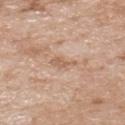<lesion>
<biopsy_status>not biopsied; imaged during a skin examination</biopsy_status>
<lighting>white-light</lighting>
<lesion_size>
  <long_diameter_mm_approx>3.0</long_diameter_mm_approx>
</lesion_size>
<site>upper back</site>
<image>
  <source>total-body photography crop</source>
  <field_of_view_mm>15</field_of_view_mm>
</image>
<patient>
  <sex>male</sex>
  <age_approx>80</age_approx>
</patient>
</lesion>